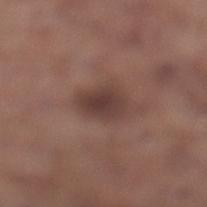No biopsy was performed on this lesion — it was imaged during a full skin examination and was not determined to be concerning.
The tile uses white-light illumination.
A close-up tile cropped from a whole-body skin photograph, about 15 mm across.
The lesion's longest dimension is about 4 mm.
A male patient aged around 55.
The lesion is located on the left lower leg.